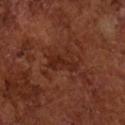Part of a total-body skin-imaging series; this lesion was reviewed on a skin check and was not flagged for biopsy. A close-up tile cropped from a whole-body skin photograph, about 15 mm across. The lesion is located on the left arm. Captured under cross-polarized illumination. A male patient in their mid-60s. Automated tile analysis of the lesion measured an area of roughly 6.5 mm², an outline eccentricity of about 0.85 (0 = round, 1 = elongated), and two-axis asymmetry of about 0.35. And it measured a mean CIELAB color near L≈25 a*≈23 b*≈26 and a normalized border contrast of about 6.5. The software also gave a border-irregularity rating of about 4/10, a color-variation rating of about 2/10, and radial color variation of about 0.5.